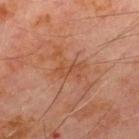Recorded during total-body skin imaging; not selected for excision or biopsy. Captured under cross-polarized illumination. Cropped from a whole-body photographic skin survey; the tile spans about 15 mm. The lesion is on the chest. Measured at roughly 3 mm in maximum diameter. A male patient about 70 years old.